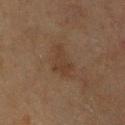Q: Automated lesion metrics?
A: an area of roughly 9 mm², an outline eccentricity of about 0.8 (0 = round, 1 = elongated), and a shape-asymmetry score of about 0.35 (0 = symmetric); a mean CIELAB color near L≈31 a*≈13 b*≈24, a lesion–skin lightness drop of about 5, and a normalized lesion–skin contrast near 5; an automated nevus-likeness rating near 0 out of 100 and a detector confidence of about 100 out of 100 that the crop contains a lesion
Q: What kind of image is this?
A: ~15 mm tile from a whole-body skin photo
Q: How large is the lesion?
A: ~4.5 mm (longest diameter)
Q: What are the patient's age and sex?
A: male, roughly 70 years of age
Q: What is the anatomic site?
A: the chest